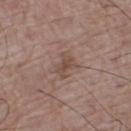Context:
The lesion's longest dimension is about 3.5 mm. A region of skin cropped from a whole-body photographic capture, roughly 15 mm wide. Captured under white-light illumination. A male patient, aged approximately 70. On the left lower leg.